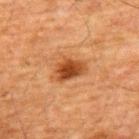Findings:
– workup: catalogued during a skin exam; not biopsied
– acquisition: ~15 mm crop, total-body skin-cancer survey
– anatomic site: the upper back
– subject: male, approximately 60 years of age
– TBP lesion metrics: a lesion area of about 8 mm², an eccentricity of roughly 0.7, and a shape-asymmetry score of about 0.3 (0 = symmetric); about 11 CIELAB-L* units darker than the surrounding skin and a normalized border contrast of about 9.5; a classifier nevus-likeness of about 95/100 and a detector confidence of about 100 out of 100 that the crop contains a lesion
– lesion diameter: ≈4 mm